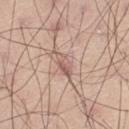* site: the left thigh
* patient: male, in their mid- to late 40s
* size: ≈3 mm
* acquisition: ~15 mm crop, total-body skin-cancer survey
* lighting: white-light illumination